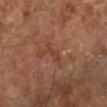A roughly 15 mm field-of-view crop from a total-body skin photograph. This is a cross-polarized tile. The patient is aged 63 to 67. The recorded lesion diameter is about 3 mm. The lesion is on the left lower leg. Automated tile analysis of the lesion measured an area of roughly 3 mm² and two-axis asymmetry of about 0.4. It also reported an average lesion color of about L≈40 a*≈23 b*≈27 (CIELAB), about 6 CIELAB-L* units darker than the surrounding skin, and a normalized lesion–skin contrast near 5.